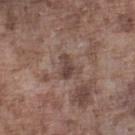– follow-up · no biopsy performed (imaged during a skin exam)
– imaging modality · ~15 mm tile from a whole-body skin photo
– tile lighting · white-light illumination
– body site · the left lower leg
– patient · male, in their mid-70s
– diameter · ≈3 mm